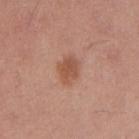The lesion was tiled from a total-body skin photograph and was not biopsied.
The subject is a female aged 23–27.
This is a white-light tile.
A region of skin cropped from a whole-body photographic capture, roughly 15 mm wide.
On the right upper arm.
About 3.5 mm across.